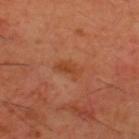follow-up: total-body-photography surveillance lesion; no biopsy | lighting: cross-polarized | anatomic site: the back | patient: male, aged around 60 | size: about 3 mm | imaging modality: ~15 mm tile from a whole-body skin photo.